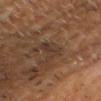Findings:
* follow-up: total-body-photography surveillance lesion; no biopsy
* imaging modality: 15 mm crop, total-body photography
* body site: the head or neck
* patient: male, about 60 years old
* size: ≈3 mm
* tile lighting: cross-polarized illumination